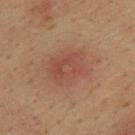The subject is a male about 35 years old. The lesion is on the back. Captured under cross-polarized illumination. Measured at roughly 5 mm in maximum diameter. The total-body-photography lesion software estimated a footprint of about 15 mm², a shape eccentricity near 0.6, and a shape-asymmetry score of about 0.2 (0 = symmetric). It also reported a mean CIELAB color near L≈43 a*≈21 b*≈26, a lesion–skin lightness drop of about 6, and a lesion-to-skin contrast of about 5 (normalized; higher = more distinct). The analysis additionally found a classifier nevus-likeness of about 0/100 and a detector confidence of about 100 out of 100 that the crop contains a lesion. A region of skin cropped from a whole-body photographic capture, roughly 15 mm wide.